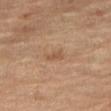notes: imaged on a skin check; not biopsied | anatomic site: the right thigh | automated lesion analysis: an area of roughly 2.5 mm², an eccentricity of roughly 0.85, and a symmetry-axis asymmetry near 0.4; a lesion-to-skin contrast of about 5.5 (normalized; higher = more distinct); a border-irregularity rating of about 4/10, a color-variation rating of about 0/10, and radial color variation of about 0 | size: ~2.5 mm (longest diameter) | acquisition: 15 mm crop, total-body photography | patient: female, in their 70s.